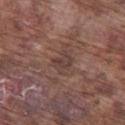Recorded during total-body skin imaging; not selected for excision or biopsy.
The tile uses white-light illumination.
Approximately 2.5 mm at its widest.
Cropped from a total-body skin-imaging series; the visible field is about 15 mm.
The lesion is located on the left thigh.
A male subject roughly 75 years of age.